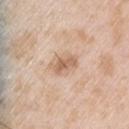follow-up: no biopsy performed (imaged during a skin exam)
image source: total-body-photography crop, ~15 mm field of view
subject: male, aged around 50
site: the left upper arm